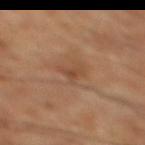No biopsy was performed on this lesion — it was imaged during a full skin examination and was not determined to be concerning.
Measured at roughly 2.5 mm in maximum diameter.
The subject is a male aged approximately 65.
A roughly 15 mm field-of-view crop from a total-body skin photograph.
The lesion is located on the right lower leg.
Automated tile analysis of the lesion measured a lesion area of about 3 mm², an outline eccentricity of about 0.8 (0 = round, 1 = elongated), and a shape-asymmetry score of about 0.6 (0 = symmetric).
The tile uses cross-polarized illumination.